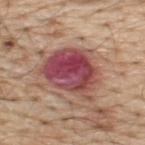Impression:
Captured during whole-body skin photography for melanoma surveillance; the lesion was not biopsied.
Image and clinical context:
Measured at roughly 7 mm in maximum diameter. The lesion is located on the back. A roughly 15 mm field-of-view crop from a total-body skin photograph. This is a white-light tile. A male subject in their 70s.